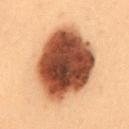Captured during whole-body skin photography for melanoma surveillance; the lesion was not biopsied. Cropped from a whole-body photographic skin survey; the tile spans about 15 mm. A female subject aged around 40. On the mid back. Automated image analysis of the tile measured a lesion area of about 48 mm² and a symmetry-axis asymmetry near 0.1. It also reported a border-irregularity index near 1.5/10 and a color-variation rating of about 8/10. Captured under cross-polarized illumination. The recorded lesion diameter is about 9.5 mm.